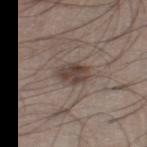This lesion was catalogued during total-body skin photography and was not selected for biopsy. An algorithmic analysis of the crop reported an area of roughly 6.5 mm² and an outline eccentricity of about 0.7 (0 = round, 1 = elongated). The analysis additionally found border irregularity of about 2.5 on a 0–10 scale, a within-lesion color-variation index near 3/10, and radial color variation of about 1. It also reported lesion-presence confidence of about 100/100. A male patient, about 20 years old. Located on the right thigh. Captured under white-light illumination. Measured at roughly 3.5 mm in maximum diameter. A 15 mm crop from a total-body photograph taken for skin-cancer surveillance.